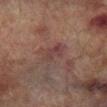follow-up: catalogued during a skin exam; not biopsied
diameter: about 3 mm
TBP lesion metrics: an automated nevus-likeness rating near 0 out of 100 and a lesion-detection confidence of about 100/100
acquisition: ~15 mm crop, total-body skin-cancer survey
anatomic site: the leg
tile lighting: cross-polarized
patient: male, roughly 75 years of age